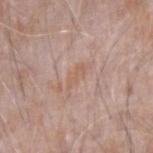Q: Was a biopsy performed?
A: catalogued during a skin exam; not biopsied
Q: How was the tile lit?
A: white-light illumination
Q: What is the lesion's diameter?
A: about 4 mm
Q: Lesion location?
A: the right forearm
Q: What kind of image is this?
A: total-body-photography crop, ~15 mm field of view
Q: Who is the patient?
A: male, about 75 years old